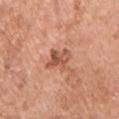This lesion was catalogued during total-body skin photography and was not selected for biopsy. The total-body-photography lesion software estimated a footprint of about 6 mm², an outline eccentricity of about 0.7 (0 = round, 1 = elongated), and two-axis asymmetry of about 0.35. It also reported a nevus-likeness score of about 10/100 and a lesion-detection confidence of about 100/100. The recorded lesion diameter is about 3 mm. A 15 mm crop from a total-body photograph taken for skin-cancer surveillance. The lesion is located on the chest. A male subject aged approximately 55.